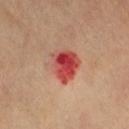Notes:
• biopsy status: imaged on a skin check; not biopsied
• patient: female, aged around 60
• size: ~4 mm (longest diameter)
• body site: the leg
• image: ~15 mm crop, total-body skin-cancer survey
• automated metrics: a footprint of about 11 mm² and a shape eccentricity near 0.55; a mean CIELAB color near L≈50 a*≈38 b*≈30, about 14 CIELAB-L* units darker than the surrounding skin, and a lesion-to-skin contrast of about 10 (normalized; higher = more distinct); a color-variation rating of about 10/10 and a peripheral color-asymmetry measure near 3.5; a detector confidence of about 100 out of 100 that the crop contains a lesion
• illumination: cross-polarized illumination